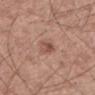Part of a total-body skin-imaging series; this lesion was reviewed on a skin check and was not flagged for biopsy.
A male patient, approximately 60 years of age.
From the mid back.
Imaged with white-light lighting.
Longest diameter approximately 3 mm.
A 15 mm crop from a total-body photograph taken for skin-cancer surveillance.